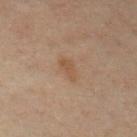Located on the front of the torso.
Imaged with cross-polarized lighting.
The patient is a male in their 40s.
A 15 mm crop from a total-body photograph taken for skin-cancer surveillance.
An algorithmic analysis of the crop reported roughly 5 lightness units darker than nearby skin and a lesion-to-skin contrast of about 5.5 (normalized; higher = more distinct).